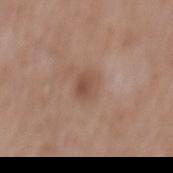Assessment: Captured during whole-body skin photography for melanoma surveillance; the lesion was not biopsied. Image and clinical context: Located on the mid back. This image is a 15 mm lesion crop taken from a total-body photograph. The patient is a male in their 60s.